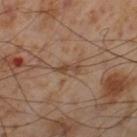Clinical impression: Part of a total-body skin-imaging series; this lesion was reviewed on a skin check and was not flagged for biopsy. Context: This is a cross-polarized tile. From the left thigh. A male patient, aged 53 to 57. Automated image analysis of the tile measured a border-irregularity index near 4/10, a color-variation rating of about 0/10, and radial color variation of about 0. Cropped from a total-body skin-imaging series; the visible field is about 15 mm.